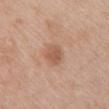| feature | finding |
|---|---|
| lighting | white-light illumination |
| image source | ~15 mm tile from a whole-body skin photo |
| lesion diameter | ≈2.5 mm |
| patient | female, aged approximately 50 |
| location | the chest |